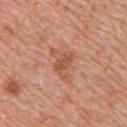workup=catalogued during a skin exam; not biopsied
subject=female, aged 38 to 42
acquisition=~15 mm crop, total-body skin-cancer survey
location=the upper back
automated lesion analysis=an automated nevus-likeness rating near 0 out of 100 and lesion-presence confidence of about 100/100
tile lighting=white-light
size=≈4.5 mm